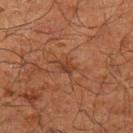Recorded during total-body skin imaging; not selected for excision or biopsy.
The patient is a male approximately 70 years of age.
A 15 mm close-up extracted from a 3D total-body photography capture.
The lesion is on the left upper arm.